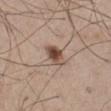The lesion was tiled from a total-body skin photograph and was not biopsied. Cropped from a total-body skin-imaging series; the visible field is about 15 mm. The lesion is on the abdomen. The lesion's longest dimension is about 2.5 mm. A male patient approximately 75 years of age.